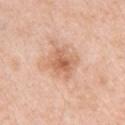Clinical impression:
Captured during whole-body skin photography for melanoma surveillance; the lesion was not biopsied.
Background:
A 15 mm close-up tile from a total-body photography series done for melanoma screening. Approximately 4 mm at its widest. On the left upper arm. Captured under white-light illumination. A male patient roughly 70 years of age.